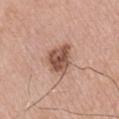* follow-up · imaged on a skin check; not biopsied
* location · the left upper arm
* lighting · white-light illumination
* image source · total-body-photography crop, ~15 mm field of view
* subject · male, in their mid- to late 70s
* image-analysis metrics · a lesion area of about 10 mm², a shape eccentricity near 0.65, and a shape-asymmetry score of about 0.25 (0 = symmetric); a border-irregularity index near 2.5/10, a within-lesion color-variation index near 4.5/10, and a peripheral color-asymmetry measure near 1.5; an automated nevus-likeness rating near 55 out of 100 and a detector confidence of about 100 out of 100 that the crop contains a lesion